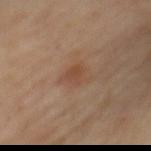Impression: This lesion was catalogued during total-body skin photography and was not selected for biopsy. Acquisition and patient details: Imaged with cross-polarized lighting. A close-up tile cropped from a whole-body skin photograph, about 15 mm across. The lesion is located on the mid back. Approximately 3 mm at its widest. A female subject roughly 65 years of age. The lesion-visualizer software estimated an area of roughly 5 mm², a shape eccentricity near 0.6, and a shape-asymmetry score of about 0.35 (0 = symmetric). It also reported an average lesion color of about L≈45 a*≈19 b*≈29 (CIELAB).